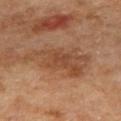{
  "biopsy_status": "not biopsied; imaged during a skin examination",
  "lighting": "cross-polarized",
  "site": "back",
  "lesion_size": {
    "long_diameter_mm_approx": 7.5
  },
  "automated_metrics": {
    "vs_skin_darker_L": 8.0,
    "vs_skin_contrast_norm": 6.5
  },
  "image": {
    "source": "total-body photography crop",
    "field_of_view_mm": 15
  },
  "patient": {
    "sex": "female",
    "age_approx": 60
  }
}